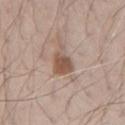Impression: The lesion was photographed on a routine skin check and not biopsied; there is no pathology result. Image and clinical context: The total-body-photography lesion software estimated a lesion area of about 7 mm², an outline eccentricity of about 0.85 (0 = round, 1 = elongated), and two-axis asymmetry of about 0.4. The software also gave a mean CIELAB color near L≈53 a*≈17 b*≈26, a lesion–skin lightness drop of about 11, and a lesion-to-skin contrast of about 8 (normalized; higher = more distinct). It also reported border irregularity of about 4.5 on a 0–10 scale and a peripheral color-asymmetry measure near 1. The analysis additionally found a classifier nevus-likeness of about 90/100. On the arm. A male patient roughly 70 years of age. Approximately 4.5 mm at its widest. This is a white-light tile. A 15 mm crop from a total-body photograph taken for skin-cancer surveillance.